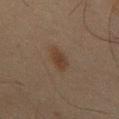Case summary:
* workup · catalogued during a skin exam; not biopsied